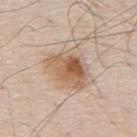Assessment: The lesion was tiled from a total-body skin photograph and was not biopsied. Context: About 5 mm across. The lesion is located on the upper back. Imaged with white-light lighting. The total-body-photography lesion software estimated a lesion area of about 17 mm², an outline eccentricity of about 0.6 (0 = round, 1 = elongated), and two-axis asymmetry of about 0.2. The software also gave an automated nevus-likeness rating near 90 out of 100 and a detector confidence of about 100 out of 100 that the crop contains a lesion. Cropped from a whole-body photographic skin survey; the tile spans about 15 mm. A male subject in their 80s.